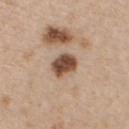Impression: Imaged during a routine full-body skin examination; the lesion was not biopsied and no histopathology is available.